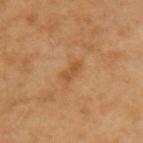Imaged during a routine full-body skin examination; the lesion was not biopsied and no histopathology is available.